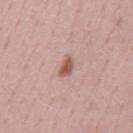– workup · imaged on a skin check; not biopsied
– size · ~3 mm (longest diameter)
– site · the mid back
– lighting · white-light illumination
– image · total-body-photography crop, ~15 mm field of view
– patient · male, aged around 50
– TBP lesion metrics · a nevus-likeness score of about 100/100 and lesion-presence confidence of about 100/100